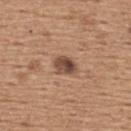Case summary:
* workup: imaged on a skin check; not biopsied
* imaging modality: 15 mm crop, total-body photography
* patient: male, roughly 65 years of age
* lesion size: ~3 mm (longest diameter)
* location: the upper back
* tile lighting: white-light illumination